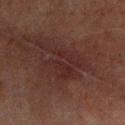Assessment:
This lesion was catalogued during total-body skin photography and was not selected for biopsy.
Image and clinical context:
The lesion-visualizer software estimated a footprint of about 14 mm², an outline eccentricity of about 0.7 (0 = round, 1 = elongated), and a symmetry-axis asymmetry near 0.45. The analysis additionally found an average lesion color of about L≈20 a*≈17 b*≈16 (CIELAB) and a lesion-to-skin contrast of about 6 (normalized; higher = more distinct). The analysis additionally found an automated nevus-likeness rating near 0 out of 100 and lesion-presence confidence of about 90/100. The tile uses cross-polarized illumination. This image is a 15 mm lesion crop taken from a total-body photograph. Located on the left lower leg. The patient is a male aged 63–67. The recorded lesion diameter is about 6 mm.